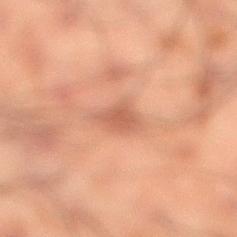{
  "site": "right lower leg",
  "patient": {
    "sex": "male",
    "age_approx": 50
  },
  "image": {
    "source": "total-body photography crop",
    "field_of_view_mm": 15
  },
  "lesion_size": {
    "long_diameter_mm_approx": 3.0
  },
  "lighting": "cross-polarized"
}